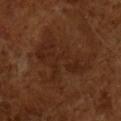<tbp_lesion>
<biopsy_status>not biopsied; imaged during a skin examination</biopsy_status>
<lesion_size>
  <long_diameter_mm_approx>8.0</long_diameter_mm_approx>
</lesion_size>
<image>
  <source>total-body photography crop</source>
  <field_of_view_mm>15</field_of_view_mm>
</image>
<patient>
  <sex>male</sex>
  <age_approx>65</age_approx>
</patient>
<automated_metrics>
  <nevus_likeness_0_100>0</nevus_likeness_0_100>
  <lesion_detection_confidence_0_100>100</lesion_detection_confidence_0_100>
</automated_metrics>
</tbp_lesion>